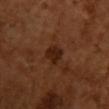Q: Is there a histopathology result?
A: total-body-photography surveillance lesion; no biopsy
Q: Lesion location?
A: the chest
Q: Lesion size?
A: ≈2.5 mm
Q: Illumination type?
A: cross-polarized illumination
Q: Who is the patient?
A: female, about 55 years old
Q: Automated lesion metrics?
A: a shape-asymmetry score of about 0.2 (0 = symmetric); an average lesion color of about L≈23 a*≈21 b*≈27 (CIELAB), a lesion–skin lightness drop of about 8, and a lesion-to-skin contrast of about 9 (normalized; higher = more distinct)
Q: What is the imaging modality?
A: total-body-photography crop, ~15 mm field of view